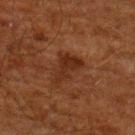Assessment:
Part of a total-body skin-imaging series; this lesion was reviewed on a skin check and was not flagged for biopsy.
Acquisition and patient details:
The tile uses cross-polarized illumination. On the upper back. A male patient, aged 58–62. A close-up tile cropped from a whole-body skin photograph, about 15 mm across.